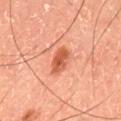biopsy status: total-body-photography surveillance lesion; no biopsy | automated metrics: an area of roughly 5.5 mm² and an outline eccentricity of about 0.8 (0 = round, 1 = elongated); a border-irregularity rating of about 2/10, a color-variation rating of about 4/10, and a peripheral color-asymmetry measure near 1 | image: total-body-photography crop, ~15 mm field of view | site: the upper back | lighting: cross-polarized illumination | subject: male, about 45 years old | size: ~3.5 mm (longest diameter).